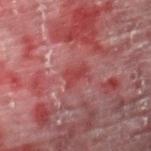No biopsy was performed on this lesion — it was imaged during a full skin examination and was not determined to be concerning. Measured at roughly 3 mm in maximum diameter. The patient is a male approximately 55 years of age. The lesion is located on the right thigh. A 15 mm close-up tile from a total-body photography series done for melanoma screening.